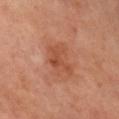Impression:
The lesion was photographed on a routine skin check and not biopsied; there is no pathology result.
Context:
This image is a 15 mm lesion crop taken from a total-body photograph. The subject is a male aged around 70. Located on the right upper arm.